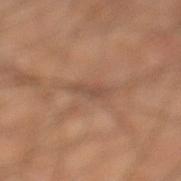Q: Was this lesion biopsied?
A: total-body-photography surveillance lesion; no biopsy
Q: Lesion location?
A: the left lower leg
Q: What lighting was used for the tile?
A: cross-polarized
Q: What kind of image is this?
A: ~15 mm tile from a whole-body skin photo
Q: What are the patient's age and sex?
A: male, roughly 60 years of age
Q: Lesion size?
A: ~3 mm (longest diameter)